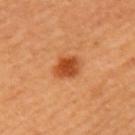Part of a total-body skin-imaging series; this lesion was reviewed on a skin check and was not flagged for biopsy. The patient is a female in their mid-40s. The lesion's longest dimension is about 3 mm. The lesion is located on the left upper arm. The total-body-photography lesion software estimated an area of roughly 7 mm², an outline eccentricity of about 0.6 (0 = round, 1 = elongated), and a symmetry-axis asymmetry near 0.2. The software also gave an average lesion color of about L≈49 a*≈32 b*≈44 (CIELAB), a lesion–skin lightness drop of about 13, and a lesion-to-skin contrast of about 9 (normalized; higher = more distinct). A lesion tile, about 15 mm wide, cut from a 3D total-body photograph. The tile uses cross-polarized illumination.